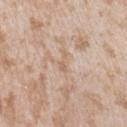| field | value |
|---|---|
| notes | catalogued during a skin exam; not biopsied |
| tile lighting | white-light illumination |
| body site | the left upper arm |
| image | ~15 mm crop, total-body skin-cancer survey |
| automated lesion analysis | a mean CIELAB color near L≈63 a*≈16 b*≈30, roughly 7 lightness units darker than nearby skin, and a normalized lesion–skin contrast near 5; a border-irregularity rating of about 6/10 |
| subject | female, aged approximately 25 |
| lesion size | about 3 mm |